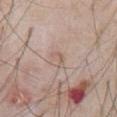This lesion was catalogued during total-body skin photography and was not selected for biopsy. This is a white-light tile. Longest diameter approximately 2.5 mm. A roughly 15 mm field-of-view crop from a total-body skin photograph. A male subject about 60 years old. Located on the abdomen. Automated tile analysis of the lesion measured border irregularity of about 6.5 on a 0–10 scale, a color-variation rating of about 0/10, and radial color variation of about 0. It also reported a nevus-likeness score of about 0/100 and a detector confidence of about 95 out of 100 that the crop contains a lesion.